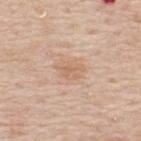No biopsy was performed on this lesion — it was imaged during a full skin examination and was not determined to be concerning.
From the upper back.
An algorithmic analysis of the crop reported a normalized lesion–skin contrast near 5. The analysis additionally found a border-irregularity rating of about 3/10, internal color variation of about 2.5 on a 0–10 scale, and a peripheral color-asymmetry measure near 1. And it measured a classifier nevus-likeness of about 0/100 and a detector confidence of about 100 out of 100 that the crop contains a lesion.
A roughly 15 mm field-of-view crop from a total-body skin photograph.
A male subject approximately 60 years of age.